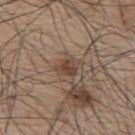{"biopsy_status": "not biopsied; imaged during a skin examination", "image": {"source": "total-body photography crop", "field_of_view_mm": 15}, "site": "mid back", "lesion_size": {"long_diameter_mm_approx": 2.5}, "patient": {"sex": "male", "age_approx": 75}, "lighting": "white-light", "automated_metrics": {"vs_skin_darker_L": 9.0, "vs_skin_contrast_norm": 7.5, "border_irregularity_0_10": 3.0, "peripheral_color_asymmetry": 1.0, "nevus_likeness_0_100": 65}}